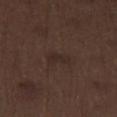Clinical impression:
The lesion was tiled from a total-body skin photograph and was not biopsied.
Image and clinical context:
The lesion is on the left thigh. Longest diameter approximately 2.5 mm. A lesion tile, about 15 mm wide, cut from a 3D total-body photograph. The patient is a male aged 68–72.